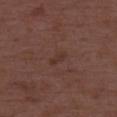Clinical impression: The lesion was tiled from a total-body skin photograph and was not biopsied. Background: A male patient, in their 50s. Measured at roughly 2.5 mm in maximum diameter. This image is a 15 mm lesion crop taken from a total-body photograph.